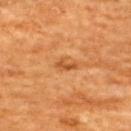Assessment:
Recorded during total-body skin imaging; not selected for excision or biopsy.
Acquisition and patient details:
From the upper back. The lesion-visualizer software estimated an outline eccentricity of about 0.9 (0 = round, 1 = elongated) and a symmetry-axis asymmetry near 0.4. It also reported a lesion color around L≈49 a*≈26 b*≈42 in CIELAB and about 9 CIELAB-L* units darker than the surrounding skin. The analysis additionally found a color-variation rating of about 0/10 and radial color variation of about 0. A lesion tile, about 15 mm wide, cut from a 3D total-body photograph. A male patient about 60 years old. The lesion's longest dimension is about 2.5 mm. The tile uses cross-polarized illumination.